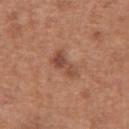Clinical impression: No biopsy was performed on this lesion — it was imaged during a full skin examination and was not determined to be concerning. Context: The patient is a male aged approximately 65. A 15 mm close-up extracted from a 3D total-body photography capture. Automated tile analysis of the lesion measured a lesion area of about 5.5 mm², an outline eccentricity of about 0.9 (0 = round, 1 = elongated), and a shape-asymmetry score of about 0.4 (0 = symmetric). And it measured roughly 9 lightness units darker than nearby skin and a lesion-to-skin contrast of about 7 (normalized; higher = more distinct). And it measured a classifier nevus-likeness of about 20/100 and a lesion-detection confidence of about 100/100. The lesion is on the abdomen. The lesion's longest dimension is about 4 mm.